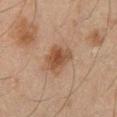Notes:
– workup · total-body-photography surveillance lesion; no biopsy
– acquisition · ~15 mm tile from a whole-body skin photo
– body site · the left lower leg
– patient · male, approximately 45 years of age